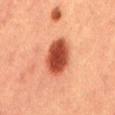The lesion was photographed on a routine skin check and not biopsied; there is no pathology result. A male subject, aged around 40. A 15 mm close-up extracted from a 3D total-body photography capture. Longest diameter approximately 5 mm. On the mid back. This is a cross-polarized tile.